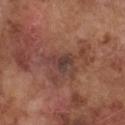Impression:
Captured during whole-body skin photography for melanoma surveillance; the lesion was not biopsied.
Clinical summary:
A male subject, in their mid- to late 70s. A 15 mm crop from a total-body photograph taken for skin-cancer surveillance. Measured at roughly 2.5 mm in maximum diameter. From the chest. This is a white-light tile.